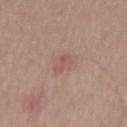| feature | finding |
|---|---|
| workup | imaged on a skin check; not biopsied |
| image-analysis metrics | a lesion color around L≈54 a*≈20 b*≈24 in CIELAB and a lesion–skin lightness drop of about 7; a border-irregularity index near 3/10 and radial color variation of about 0 |
| body site | the mid back |
| illumination | white-light illumination |
| lesion size | ~3 mm (longest diameter) |
| imaging modality | 15 mm crop, total-body photography |
| patient | male, approximately 55 years of age |